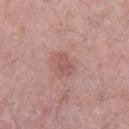biopsy status=catalogued during a skin exam; not biopsied | tile lighting=white-light illumination | body site=the upper back | acquisition=~15 mm crop, total-body skin-cancer survey | patient=male, roughly 25 years of age | image-analysis metrics=a lesion color around L≈54 a*≈23 b*≈23 in CIELAB and about 8 CIELAB-L* units darker than the surrounding skin; a border-irregularity rating of about 3/10, internal color variation of about 2 on a 0–10 scale, and a peripheral color-asymmetry measure near 1 | diameter=about 3 mm.